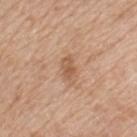Assessment: No biopsy was performed on this lesion — it was imaged during a full skin examination and was not determined to be concerning. Background: The lesion's longest dimension is about 2.5 mm. The lesion-visualizer software estimated a shape-asymmetry score of about 0.25 (0 = symmetric). The software also gave about 9 CIELAB-L* units darker than the surrounding skin. And it measured a detector confidence of about 100 out of 100 that the crop contains a lesion. From the upper back. A female patient, in their mid-50s. Imaged with white-light lighting. Cropped from a total-body skin-imaging series; the visible field is about 15 mm.